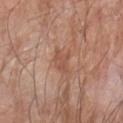  biopsy_status: not biopsied; imaged during a skin examination
  patient:
    sex: male
    age_approx: 60
  image:
    source: total-body photography crop
    field_of_view_mm: 15
  site: arm